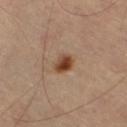Notes:
• notes · no biopsy performed (imaged during a skin exam)
• patient · male, aged approximately 65
• TBP lesion metrics · a mean CIELAB color near L≈42 a*≈21 b*≈31 and a normalized lesion–skin contrast near 11
• diameter · about 2.5 mm
• acquisition · total-body-photography crop, ~15 mm field of view
• tile lighting · cross-polarized
• site · the right thigh